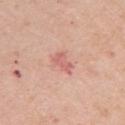Impression: Imaged during a routine full-body skin examination; the lesion was not biopsied and no histopathology is available. Background: A female subject, roughly 65 years of age. On the left upper arm. A 15 mm crop from a total-body photograph taken for skin-cancer surveillance.